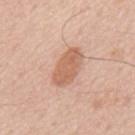This lesion was catalogued during total-body skin photography and was not selected for biopsy. The total-body-photography lesion software estimated an average lesion color of about L≈62 a*≈22 b*≈32 (CIELAB) and a lesion–skin lightness drop of about 10. A roughly 15 mm field-of-view crop from a total-body skin photograph. The lesion is located on the mid back. About 5 mm across. This is a white-light tile. The patient is a male roughly 65 years of age.